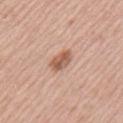biopsy status: catalogued during a skin exam; not biopsied
patient: male, aged 53 to 57
TBP lesion metrics: a lesion area of about 5 mm², a shape eccentricity near 0.85, and a shape-asymmetry score of about 0.2 (0 = symmetric); a border-irregularity index near 2.5/10 and radial color variation of about 1
location: the arm
image: ~15 mm tile from a whole-body skin photo
lesion size: about 3.5 mm